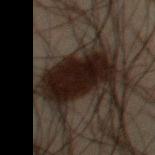The lesion was tiled from a total-body skin photograph and was not biopsied.
A region of skin cropped from a whole-body photographic capture, roughly 15 mm wide.
A male subject aged approximately 50.
On the right thigh.
About 6 mm across.